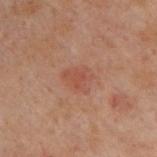Q: Was a biopsy performed?
A: total-body-photography surveillance lesion; no biopsy
Q: How was the tile lit?
A: cross-polarized illumination
Q: Automated lesion metrics?
A: a footprint of about 7 mm², an outline eccentricity of about 0.55 (0 = round, 1 = elongated), and a shape-asymmetry score of about 0.35 (0 = symmetric); a mean CIELAB color near L≈41 a*≈20 b*≈25 and roughly 5 lightness units darker than nearby skin; a border-irregularity rating of about 3/10 and internal color variation of about 2 on a 0–10 scale
Q: What are the patient's age and sex?
A: male, aged 48 to 52
Q: How large is the lesion?
A: ~3.5 mm (longest diameter)
Q: What is the anatomic site?
A: the upper back
Q: What is the imaging modality?
A: ~15 mm tile from a whole-body skin photo